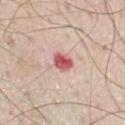| feature | finding |
|---|---|
| follow-up | catalogued during a skin exam; not biopsied |
| body site | the chest |
| acquisition | ~15 mm tile from a whole-body skin photo |
| size | about 2.5 mm |
| patient | male, aged 78 to 82 |